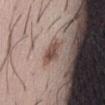Captured during whole-body skin photography for melanoma surveillance; the lesion was not biopsied. A 15 mm close-up tile from a total-body photography series done for melanoma screening. Located on the front of the torso. Longest diameter approximately 3.5 mm. The patient is a male aged around 30. The total-body-photography lesion software estimated border irregularity of about 3.5 on a 0–10 scale and a within-lesion color-variation index near 2.5/10. It also reported a classifier nevus-likeness of about 0/100 and a lesion-detection confidence of about 100/100.